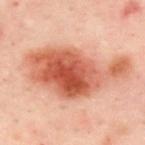No biopsy was performed on this lesion — it was imaged during a full skin examination and was not determined to be concerning.
A 15 mm close-up tile from a total-body photography series done for melanoma screening.
A female patient, aged approximately 40.
From the upper back.
Longest diameter approximately 11 mm.